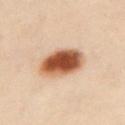No biopsy was performed on this lesion — it was imaged during a full skin examination and was not determined to be concerning. A female patient, approximately 60 years of age. Cropped from a whole-body photographic skin survey; the tile spans about 15 mm. Located on the chest. This is a cross-polarized tile. About 5 mm across.